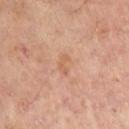follow-up = imaged on a skin check; not biopsied | lesion diameter = ~2.5 mm (longest diameter) | tile lighting = cross-polarized illumination | imaging modality = 15 mm crop, total-body photography | subject = aged around 55 | site = the right upper arm | image-analysis metrics = a nevus-likeness score of about 0/100.